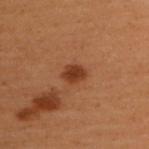{"patient": {"sex": "female", "age_approx": 50}, "image": {"source": "total-body photography crop", "field_of_view_mm": 15}, "site": "upper back"}